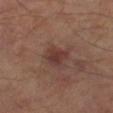| feature | finding |
|---|---|
| notes | total-body-photography surveillance lesion; no biopsy |
| lesion diameter | ~3 mm (longest diameter) |
| body site | the left lower leg |
| tile lighting | cross-polarized illumination |
| imaging modality | total-body-photography crop, ~15 mm field of view |
| patient | in their mid- to late 60s |